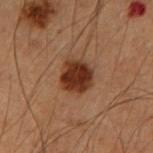  site: right forearm
  lesion_size:
    long_diameter_mm_approx: 3.5
  patient:
    sex: male
    age_approx: 55
  image:
    source: total-body photography crop
    field_of_view_mm: 15